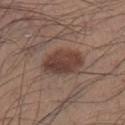follow-up: no biopsy performed (imaged during a skin exam) | acquisition: ~15 mm tile from a whole-body skin photo | patient: male, aged 33–37 | body site: the left lower leg.